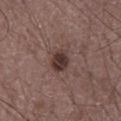Captured during whole-body skin photography for melanoma surveillance; the lesion was not biopsied.
A 15 mm crop from a total-body photograph taken for skin-cancer surveillance.
The lesion is located on the chest.
The subject is a male aged approximately 60.